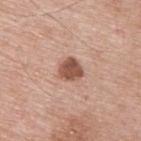Findings:
– follow-up · total-body-photography surveillance lesion; no biopsy
– subject · male, aged around 65
– illumination · white-light
– size · ≈3 mm
– body site · the upper back
– imaging modality · total-body-photography crop, ~15 mm field of view